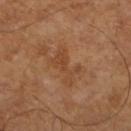biopsy status = total-body-photography surveillance lesion; no biopsy | diameter = about 5 mm | imaging modality = 15 mm crop, total-body photography | subject = male, in their mid-60s | automated metrics = an eccentricity of roughly 0.9 and a symmetry-axis asymmetry near 0.55; a nevus-likeness score of about 0/100 and lesion-presence confidence of about 100/100 | anatomic site = the left lower leg.